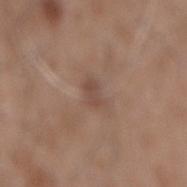Q: Was this lesion biopsied?
A: catalogued during a skin exam; not biopsied
Q: Illumination type?
A: white-light illumination
Q: What is the imaging modality?
A: ~15 mm tile from a whole-body skin photo
Q: Who is the patient?
A: male, aged approximately 60
Q: Lesion size?
A: about 2.5 mm
Q: Where on the body is the lesion?
A: the mid back